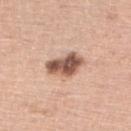Q: Was a biopsy performed?
A: imaged on a skin check; not biopsied
Q: What lighting was used for the tile?
A: white-light
Q: Lesion size?
A: ~4.5 mm (longest diameter)
Q: Who is the patient?
A: female, aged approximately 70
Q: What is the imaging modality?
A: total-body-photography crop, ~15 mm field of view
Q: What did automated image analysis measure?
A: a mean CIELAB color near L≈55 a*≈20 b*≈28 and a lesion-to-skin contrast of about 11.5 (normalized; higher = more distinct); a classifier nevus-likeness of about 85/100 and a lesion-detection confidence of about 100/100
Q: Where on the body is the lesion?
A: the right lower leg